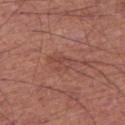Assessment: The lesion was tiled from a total-body skin photograph and was not biopsied. Background: Measured at roughly 3.5 mm in maximum diameter. This is a white-light tile. A 15 mm close-up extracted from a 3D total-body photography capture. An algorithmic analysis of the crop reported an area of roughly 4 mm² and a symmetry-axis asymmetry near 0.5. And it measured border irregularity of about 6 on a 0–10 scale, a within-lesion color-variation index near 1.5/10, and radial color variation of about 0.5. The subject is a male approximately 65 years of age. The lesion is on the right thigh.